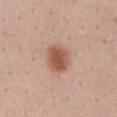Cropped from a whole-body photographic skin survey; the tile spans about 15 mm. A female subject, in their mid-30s. The total-body-photography lesion software estimated an area of roughly 8.5 mm², a shape eccentricity near 0.6, and two-axis asymmetry of about 0.2. The analysis additionally found a border-irregularity rating of about 1.5/10, internal color variation of about 4.5 on a 0–10 scale, and a peripheral color-asymmetry measure near 1.5. The analysis additionally found a classifier nevus-likeness of about 100/100 and a detector confidence of about 100 out of 100 that the crop contains a lesion. About 3.5 mm across. On the mid back.